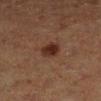automated metrics: a lesion color around L≈24 a*≈18 b*≈22 in CIELAB, about 9 CIELAB-L* units darker than the surrounding skin, and a lesion-to-skin contrast of about 10 (normalized; higher = more distinct); a border-irregularity rating of about 1.5/10, a color-variation rating of about 3/10, and peripheral color asymmetry of about 1
tile lighting: cross-polarized illumination
patient: female, aged approximately 40
location: the right lower leg
size: ~2.5 mm (longest diameter)
imaging modality: total-body-photography crop, ~15 mm field of view